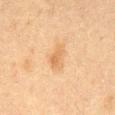From the abdomen. A male patient, in their mid-70s. Longest diameter approximately 3.5 mm. This is a cross-polarized tile. A 15 mm close-up tile from a total-body photography series done for melanoma screening.